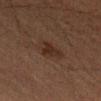{
  "biopsy_status": "not biopsied; imaged during a skin examination",
  "lesion_size": {
    "long_diameter_mm_approx": 3.0
  },
  "image": {
    "source": "total-body photography crop",
    "field_of_view_mm": 15
  },
  "lighting": "cross-polarized",
  "site": "left forearm",
  "patient": {
    "sex": "male",
    "age_approx": 50
  }
}